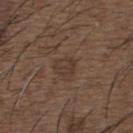Q: Is there a histopathology result?
A: total-body-photography surveillance lesion; no biopsy
Q: Where on the body is the lesion?
A: the upper back
Q: How was this image acquired?
A: ~15 mm crop, total-body skin-cancer survey
Q: Lesion size?
A: ~3 mm (longest diameter)
Q: Who is the patient?
A: male, about 50 years old